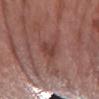Clinical impression: Recorded during total-body skin imaging; not selected for excision or biopsy. Acquisition and patient details: The lesion-visualizer software estimated a border-irregularity rating of about 2.5/10, a within-lesion color-variation index near 1.5/10, and peripheral color asymmetry of about 0.5. The software also gave a nevus-likeness score of about 5/100 and a detector confidence of about 100 out of 100 that the crop contains a lesion. Located on the arm. Longest diameter approximately 3 mm. Imaged with white-light lighting. A female patient, in their 60s. A 15 mm close-up extracted from a 3D total-body photography capture.